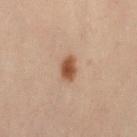Findings:
• follow-up · imaged on a skin check; not biopsied
• automated lesion analysis · a lesion–skin lightness drop of about 11 and a normalized border contrast of about 10; peripheral color asymmetry of about 0.5; a classifier nevus-likeness of about 100/100 and a detector confidence of about 100 out of 100 that the crop contains a lesion
• size · ~2.5 mm (longest diameter)
• location · the lower back
• imaging modality · total-body-photography crop, ~15 mm field of view
• illumination · cross-polarized
• subject · female, in their 30s